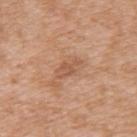{
  "biopsy_status": "not biopsied; imaged during a skin examination",
  "lighting": "white-light",
  "site": "upper back",
  "patient": {
    "sex": "female",
    "age_approx": 40
  },
  "automated_metrics": {
    "eccentricity": 0.9,
    "cielab_L": 55,
    "cielab_a": 22,
    "cielab_b": 33,
    "vs_skin_darker_L": 8.0,
    "peripheral_color_asymmetry": 0.5,
    "nevus_likeness_0_100": 0,
    "lesion_detection_confidence_0_100": 100
  },
  "image": {
    "source": "total-body photography crop",
    "field_of_view_mm": 15
  },
  "lesion_size": {
    "long_diameter_mm_approx": 3.0
  }
}